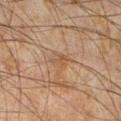– follow-up · catalogued during a skin exam; not biopsied
– tile lighting · cross-polarized
– automated metrics · a footprint of about 3 mm², an eccentricity of roughly 0.8, and a shape-asymmetry score of about 0.3 (0 = symmetric); about 6 CIELAB-L* units darker than the surrounding skin; border irregularity of about 3.5 on a 0–10 scale, internal color variation of about 1.5 on a 0–10 scale, and a peripheral color-asymmetry measure near 0.5
– patient · male, aged 43 to 47
– site · the right lower leg
– image source · ~15 mm tile from a whole-body skin photo